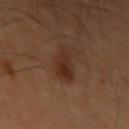Clinical impression: The lesion was tiled from a total-body skin photograph and was not biopsied. Background: Approximately 4 mm at its widest. A male subject, approximately 55 years of age. From the left upper arm. Imaged with cross-polarized lighting. This image is a 15 mm lesion crop taken from a total-body photograph.